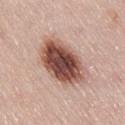<record>
  <patient>
    <sex>female</sex>
    <age_approx>50</age_approx>
  </patient>
  <site>right thigh</site>
  <lighting>white-light</lighting>
  <lesion_size>
    <long_diameter_mm_approx>7.5</long_diameter_mm_approx>
  </lesion_size>
  <image>
    <source>total-body photography crop</source>
    <field_of_view_mm>15</field_of_view_mm>
  </image>
</record>